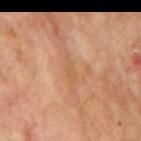– follow-up: no biopsy performed (imaged during a skin exam)